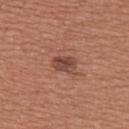The lesion was photographed on a routine skin check and not biopsied; there is no pathology result. Measured at roughly 3 mm in maximum diameter. From the upper back. A female patient, aged approximately 50. Cropped from a total-body skin-imaging series; the visible field is about 15 mm. The tile uses white-light illumination.